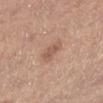Recorded during total-body skin imaging; not selected for excision or biopsy. The lesion is located on the left lower leg. The subject is a female approximately 65 years of age. The lesion's longest dimension is about 3 mm. Imaged with white-light lighting. Cropped from a whole-body photographic skin survey; the tile spans about 15 mm.